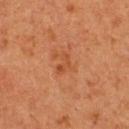On the upper back. A female patient aged around 40. Imaged with cross-polarized lighting. Cropped from a whole-body photographic skin survey; the tile spans about 15 mm. Longest diameter approximately 2.5 mm. The lesion-visualizer software estimated an area of roughly 2.5 mm², an outline eccentricity of about 0.8 (0 = round, 1 = elongated), and a symmetry-axis asymmetry near 0.55. And it measured a lesion color around L≈41 a*≈24 b*≈34 in CIELAB and a lesion-to-skin contrast of about 5.5 (normalized; higher = more distinct).